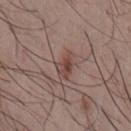{"image": {"source": "total-body photography crop", "field_of_view_mm": 15}, "site": "chest", "lesion_size": {"long_diameter_mm_approx": 3.5}, "patient": {"sex": "male", "age_approx": 55}, "automated_metrics": {"area_mm2_approx": 5.0, "eccentricity": 0.85, "shape_asymmetry": 0.35, "border_irregularity_0_10": 3.5, "color_variation_0_10": 4.5, "nevus_likeness_0_100": 45, "lesion_detection_confidence_0_100": 100}, "lighting": "white-light"}